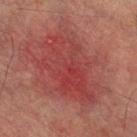The lesion was photographed on a routine skin check and not biopsied; there is no pathology result.
A male patient aged around 75.
About 9 mm across.
The total-body-photography lesion software estimated a nevus-likeness score of about 0/100.
The tile uses cross-polarized illumination.
From the right lower leg.
A close-up tile cropped from a whole-body skin photograph, about 15 mm across.